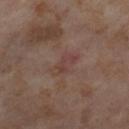<record>
  <biopsy_status>not biopsied; imaged during a skin examination</biopsy_status>
  <image>
    <source>total-body photography crop</source>
    <field_of_view_mm>15</field_of_view_mm>
  </image>
  <site>right lower leg</site>
  <patient>
    <sex>female</sex>
    <age_approx>55</age_approx>
  </patient>
  <lighting>cross-polarized</lighting>
  <automated_metrics>
    <area_mm2_approx>5.0</area_mm2_approx>
    <eccentricity>0.85</eccentricity>
    <shape_asymmetry>0.3</shape_asymmetry>
    <vs_skin_darker_L>5.0</vs_skin_darker_L>
    <vs_skin_contrast_norm>4.5</vs_skin_contrast_norm>
    <border_irregularity_0_10>3.0</border_irregularity_0_10>
    <nevus_likeness_0_100>0</nevus_likeness_0_100>
    <lesion_detection_confidence_0_100>100</lesion_detection_confidence_0_100>
  </automated_metrics>
  <lesion_size>
    <long_diameter_mm_approx>3.5</long_diameter_mm_approx>
  </lesion_size>
</record>